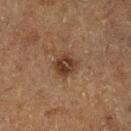| field | value |
|---|---|
| notes | catalogued during a skin exam; not biopsied |
| lighting | cross-polarized |
| image | 15 mm crop, total-body photography |
| image-analysis metrics | a border-irregularity rating of about 2/10 and radial color variation of about 1.5; a nevus-likeness score of about 80/100 and a detector confidence of about 100 out of 100 that the crop contains a lesion |
| anatomic site | the leg |
| subject | male, about 75 years old |
| diameter | about 3 mm |